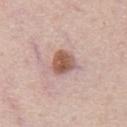Assessment:
Recorded during total-body skin imaging; not selected for excision or biopsy.
Clinical summary:
A close-up tile cropped from a whole-body skin photograph, about 15 mm across. A male patient, aged around 60. On the abdomen.